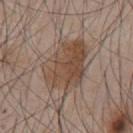Findings:
– follow-up · no biopsy performed (imaged during a skin exam)
– patient · male, aged approximately 45
– TBP lesion metrics · an average lesion color of about L≈47 a*≈15 b*≈26 (CIELAB), about 9 CIELAB-L* units darker than the surrounding skin, and a normalized lesion–skin contrast near 7.5; a border-irregularity index near 3.5/10
– imaging modality · ~15 mm crop, total-body skin-cancer survey
– lighting · white-light
– site · the chest
– diameter · ~7 mm (longest diameter)